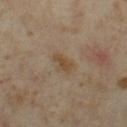Findings:
• follow-up: imaged on a skin check; not biopsied